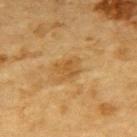– notes · total-body-photography surveillance lesion; no biopsy
– anatomic site · the upper back
– tile lighting · cross-polarized
– subject · male, aged 83 or older
– acquisition · total-body-photography crop, ~15 mm field of view
– diameter · ≈3 mm